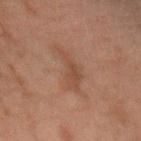Clinical impression: The lesion was tiled from a total-body skin photograph and was not biopsied. Acquisition and patient details: A close-up tile cropped from a whole-body skin photograph, about 15 mm across. The lesion is on the right forearm. A male subject, in their mid- to late 40s. The lesion's longest dimension is about 6 mm. The total-body-photography lesion software estimated an area of roughly 9 mm² and two-axis asymmetry of about 0.5. The software also gave a lesion–skin lightness drop of about 5 and a normalized border contrast of about 5.